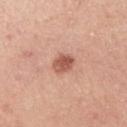Impression: No biopsy was performed on this lesion — it was imaged during a full skin examination and was not determined to be concerning. Clinical summary: This is a white-light tile. Approximately 3 mm at its widest. A female subject aged 43 to 47. A 15 mm close-up extracted from a 3D total-body photography capture. The lesion is on the right upper arm. Automated image analysis of the tile measured a footprint of about 5 mm² and an eccentricity of roughly 0.6. The software also gave a border-irregularity rating of about 2/10, internal color variation of about 3.5 on a 0–10 scale, and peripheral color asymmetry of about 1.5.